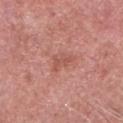<lesion>
<biopsy_status>not biopsied; imaged during a skin examination</biopsy_status>
<site>head or neck</site>
<lesion_size>
  <long_diameter_mm_approx>3.0</long_diameter_mm_approx>
</lesion_size>
<automated_metrics>
  <eccentricity>0.65</eccentricity>
  <shape_asymmetry>0.4</shape_asymmetry>
  <cielab_L>54</cielab_L>
  <cielab_a>26</cielab_a>
  <cielab_b>28</cielab_b>
  <vs_skin_darker_L>7.0</vs_skin_darker_L>
  <vs_skin_contrast_norm>5.0</vs_skin_contrast_norm>
  <border_irregularity_0_10>4.0</border_irregularity_0_10>
  <color_variation_0_10>2.5</color_variation_0_10>
  <peripheral_color_asymmetry>1.0</peripheral_color_asymmetry>
  <nevus_likeness_0_100>0</nevus_likeness_0_100>
</automated_metrics>
<image>
  <source>total-body photography crop</source>
  <field_of_view_mm>15</field_of_view_mm>
</image>
<lighting>white-light</lighting>
<patient>
  <sex>male</sex>
  <age_approx>60</age_approx>
</patient>
</lesion>